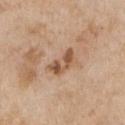The total-body-photography lesion software estimated a mean CIELAB color near L≈53 a*≈20 b*≈33, roughly 12 lightness units darker than nearby skin, and a lesion-to-skin contrast of about 8.5 (normalized; higher = more distinct). It also reported a border-irregularity rating of about 6.5/10, a color-variation rating of about 3.5/10, and radial color variation of about 1. The analysis additionally found a nevus-likeness score of about 10/100 and a lesion-detection confidence of about 100/100. Located on the chest. The tile uses white-light illumination. A female subject aged approximately 65. A 15 mm close-up tile from a total-body photography series done for melanoma screening.